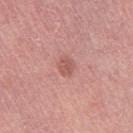No biopsy was performed on this lesion — it was imaged during a full skin examination and was not determined to be concerning.
The lesion is located on the right thigh.
The lesion-visualizer software estimated a mean CIELAB color near L≈56 a*≈26 b*≈25, a lesion–skin lightness drop of about 8, and a lesion-to-skin contrast of about 6 (normalized; higher = more distinct).
This is a white-light tile.
A lesion tile, about 15 mm wide, cut from a 3D total-body photograph.
A female patient, in their mid- to late 60s.
About 2.5 mm across.